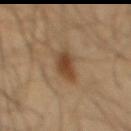notes = total-body-photography surveillance lesion; no biopsy | image = total-body-photography crop, ~15 mm field of view | location = the mid back | illumination = cross-polarized | automated lesion analysis = a lesion area of about 7 mm² and a symmetry-axis asymmetry near 0.25; border irregularity of about 3 on a 0–10 scale and internal color variation of about 3.5 on a 0–10 scale; a classifier nevus-likeness of about 95/100 and lesion-presence confidence of about 100/100 | patient = male, aged approximately 65.